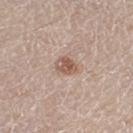<tbp_lesion>
  <biopsy_status>not biopsied; imaged during a skin examination</biopsy_status>
</tbp_lesion>